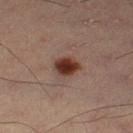<lesion>
<lesion_size>
  <long_diameter_mm_approx>3.0</long_diameter_mm_approx>
</lesion_size>
<site>leg</site>
<automated_metrics>
  <area_mm2_approx>6.0</area_mm2_approx>
  <nevus_likeness_0_100>100</nevus_likeness_0_100>
  <lesion_detection_confidence_0_100>100</lesion_detection_confidence_0_100>
</automated_metrics>
<lighting>cross-polarized</lighting>
<image>
  <source>total-body photography crop</source>
  <field_of_view_mm>15</field_of_view_mm>
</image>
<patient>
  <sex>male</sex>
  <age_approx>55</age_approx>
</patient>
</lesion>